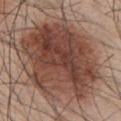{
  "biopsy_status": "not biopsied; imaged during a skin examination",
  "image": {
    "source": "total-body photography crop",
    "field_of_view_mm": 15
  },
  "site": "back",
  "lighting": "white-light",
  "lesion_size": {
    "long_diameter_mm_approx": 12.0
  },
  "patient": {
    "sex": "male",
    "age_approx": 55
  }
}